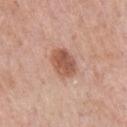{
  "biopsy_status": "not biopsied; imaged during a skin examination",
  "automated_metrics": {
    "area_mm2_approx": 8.5,
    "shape_asymmetry": 0.1,
    "cielab_L": 55,
    "cielab_a": 23,
    "cielab_b": 30,
    "vs_skin_contrast_norm": 8.5,
    "border_irregularity_0_10": 1.5,
    "color_variation_0_10": 3.5,
    "peripheral_color_asymmetry": 1.0,
    "nevus_likeness_0_100": 95,
    "lesion_detection_confidence_0_100": 100
  },
  "lighting": "white-light",
  "image": {
    "source": "total-body photography crop",
    "field_of_view_mm": 15
  },
  "site": "chest",
  "patient": {
    "sex": "male",
    "age_approx": 55
  }
}